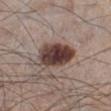Case summary:
- notes — no biopsy performed (imaged during a skin exam)
- location — the left lower leg
- imaging modality — 15 mm crop, total-body photography
- patient — male, aged approximately 60
- diameter — about 5.5 mm